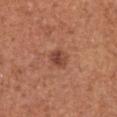The lesion was tiled from a total-body skin photograph and was not biopsied. Approximately 2.5 mm at its widest. Captured under white-light illumination. On the chest. The patient is a female aged approximately 50. A roughly 15 mm field-of-view crop from a total-body skin photograph. Automated image analysis of the tile measured a mean CIELAB color near L≈45 a*≈25 b*≈29, roughly 10 lightness units darker than nearby skin, and a normalized border contrast of about 7.5. And it measured a border-irregularity index near 1.5/10, a within-lesion color-variation index near 3.5/10, and peripheral color asymmetry of about 1.5. The analysis additionally found an automated nevus-likeness rating near 80 out of 100.